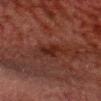  biopsy_status: not biopsied; imaged during a skin examination
  image:
    source: total-body photography crop
    field_of_view_mm: 15
  site: head or neck
  patient:
    sex: male
    age_approx: 60